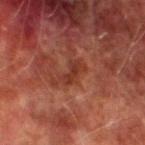Assessment:
No biopsy was performed on this lesion — it was imaged during a full skin examination and was not determined to be concerning.
Acquisition and patient details:
Imaged with cross-polarized lighting. A roughly 15 mm field-of-view crop from a total-body skin photograph. A male subject, aged approximately 75. The total-body-photography lesion software estimated an area of roughly 4.5 mm², a shape eccentricity near 0.85, and two-axis asymmetry of about 0.35. It also reported a classifier nevus-likeness of about 0/100 and lesion-presence confidence of about 100/100. Measured at roughly 3 mm in maximum diameter. Located on the leg.